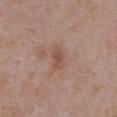| feature | finding |
|---|---|
| notes | catalogued during a skin exam; not biopsied |
| tile lighting | white-light |
| image-analysis metrics | an outline eccentricity of about 0.8 (0 = round, 1 = elongated); a mean CIELAB color near L≈51 a*≈20 b*≈26 and a normalized lesion–skin contrast near 5.5; border irregularity of about 2.5 on a 0–10 scale and internal color variation of about 2.5 on a 0–10 scale |
| size | ~3 mm (longest diameter) |
| body site | the chest |
| patient | female, approximately 35 years of age |
| acquisition | total-body-photography crop, ~15 mm field of view |